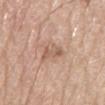Captured during whole-body skin photography for melanoma surveillance; the lesion was not biopsied. On the mid back. Longest diameter approximately 3 mm. This is a white-light tile. A male subject, in their 80s. A 15 mm close-up tile from a total-body photography series done for melanoma screening.